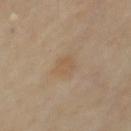Assessment: Recorded during total-body skin imaging; not selected for excision or biopsy. Image and clinical context: Imaged with cross-polarized lighting. A region of skin cropped from a whole-body photographic capture, roughly 15 mm wide. Automated tile analysis of the lesion measured a border-irregularity index near 2/10, a color-variation rating of about 1.5/10, and a peripheral color-asymmetry measure near 0.5. The software also gave an automated nevus-likeness rating near 0 out of 100 and a detector confidence of about 100 out of 100 that the crop contains a lesion. Located on the front of the torso.